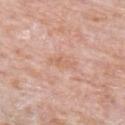This lesion was catalogued during total-body skin photography and was not selected for biopsy.
The lesion is on the right upper arm.
An algorithmic analysis of the crop reported an area of roughly 4.5 mm², a shape eccentricity near 0.85, and a symmetry-axis asymmetry near 0.35. And it measured an average lesion color of about L≈64 a*≈21 b*≈31 (CIELAB), a lesion–skin lightness drop of about 6, and a lesion-to-skin contrast of about 5 (normalized; higher = more distinct). And it measured a classifier nevus-likeness of about 0/100 and a lesion-detection confidence of about 100/100.
A 15 mm crop from a total-body photograph taken for skin-cancer surveillance.
The patient is a male approximately 80 years of age.
Captured under white-light illumination.
The lesion's longest dimension is about 3.5 mm.